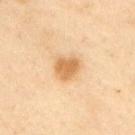Case summary:
• follow-up — imaged on a skin check; not biopsied
• location — the left upper arm
• patient — male, roughly 55 years of age
• acquisition — ~15 mm tile from a whole-body skin photo